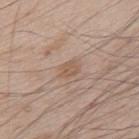<case>
<biopsy_status>not biopsied; imaged during a skin examination</biopsy_status>
<site>upper back</site>
<patient>
  <sex>male</sex>
  <age_approx>65</age_approx>
</patient>
<image>
  <source>total-body photography crop</source>
  <field_of_view_mm>15</field_of_view_mm>
</image>
<lighting>white-light</lighting>
</case>